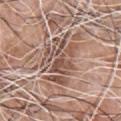The lesion was tiled from a total-body skin photograph and was not biopsied.
A region of skin cropped from a whole-body photographic capture, roughly 15 mm wide.
Captured under white-light illumination.
Measured at roughly 5.5 mm in maximum diameter.
On the chest.
A male subject aged 58 to 62.